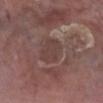<tbp_lesion>
<image>
  <source>total-body photography crop</source>
  <field_of_view_mm>15</field_of_view_mm>
</image>
<automated_metrics>
  <area_mm2_approx>8.0</area_mm2_approx>
  <eccentricity>0.7</eccentricity>
  <vs_skin_darker_L>6.0</vs_skin_darker_L>
  <vs_skin_contrast_norm>5.5</vs_skin_contrast_norm>
  <border_irregularity_0_10>1.5</border_irregularity_0_10>
  <peripheral_color_asymmetry>1.0</peripheral_color_asymmetry>
  <nevus_likeness_0_100>0</nevus_likeness_0_100>
  <lesion_detection_confidence_0_100>80</lesion_detection_confidence_0_100>
</automated_metrics>
<site>right lower leg</site>
<lighting>white-light</lighting>
<lesion_size>
  <long_diameter_mm_approx>3.5</long_diameter_mm_approx>
</lesion_size>
<patient>
  <sex>male</sex>
  <age_approx>80</age_approx>
</patient>
</tbp_lesion>